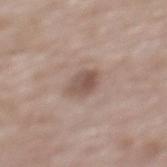Imaged during a routine full-body skin examination; the lesion was not biopsied and no histopathology is available. Cropped from a total-body skin-imaging series; the visible field is about 15 mm. Imaged with white-light lighting. The recorded lesion diameter is about 3 mm. From the upper back. A male patient, in their mid- to late 80s or older.